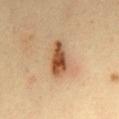workup: total-body-photography surveillance lesion; no biopsy
lesion size: ~4.5 mm (longest diameter)
patient: male, roughly 35 years of age
image source: 15 mm crop, total-body photography
TBP lesion metrics: a mean CIELAB color near L≈46 a*≈20 b*≈32 and a lesion–skin lightness drop of about 14; border irregularity of about 2.5 on a 0–10 scale and a color-variation rating of about 5.5/10
tile lighting: cross-polarized
location: the mid back